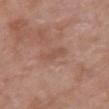Captured during whole-body skin photography for melanoma surveillance; the lesion was not biopsied. Imaged with white-light lighting. Automated tile analysis of the lesion measured an eccentricity of roughly 0.95 and a shape-asymmetry score of about 0.35 (0 = symmetric). The analysis additionally found a lesion color around L≈52 a*≈21 b*≈28 in CIELAB and a lesion-to-skin contrast of about 5 (normalized; higher = more distinct). It also reported a border-irregularity rating of about 4/10, internal color variation of about 1 on a 0–10 scale, and radial color variation of about 0. From the chest. A region of skin cropped from a whole-body photographic capture, roughly 15 mm wide. A female patient aged around 70. Longest diameter approximately 4 mm.